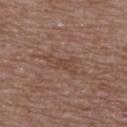Captured during whole-body skin photography for melanoma surveillance; the lesion was not biopsied. The lesion-visualizer software estimated a mean CIELAB color near L≈44 a*≈19 b*≈26 and a normalized lesion–skin contrast near 5. The software also gave border irregularity of about 6.5 on a 0–10 scale, a within-lesion color-variation index near 0.5/10, and peripheral color asymmetry of about 0. And it measured a nevus-likeness score of about 0/100. A region of skin cropped from a whole-body photographic capture, roughly 15 mm wide. The patient is a female in their mid-60s. Located on the back. The tile uses white-light illumination. About 4.5 mm across.